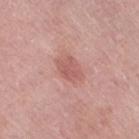Located on the right thigh. A close-up tile cropped from a whole-body skin photograph, about 15 mm across. A female subject, in their 70s.